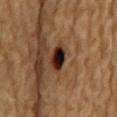Located on the back.
Automated tile analysis of the lesion measured a border-irregularity rating of about 2/10, a color-variation rating of about 10/10, and peripheral color asymmetry of about 4. And it measured a lesion-detection confidence of about 100/100.
Cropped from a whole-body photographic skin survey; the tile spans about 15 mm.
A male patient, approximately 85 years of age or older.
Approximately 3.5 mm at its widest.
Captured under cross-polarized illumination.